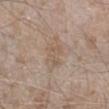The lesion was photographed on a routine skin check and not biopsied; there is no pathology result. The lesion is on the right lower leg. A male subject in their mid- to late 40s. The total-body-photography lesion software estimated border irregularity of about 4 on a 0–10 scale, a within-lesion color-variation index near 2.5/10, and radial color variation of about 1. The analysis additionally found a nevus-likeness score of about 0/100 and lesion-presence confidence of about 100/100. Approximately 4 mm at its widest. A close-up tile cropped from a whole-body skin photograph, about 15 mm across. This is a white-light tile.